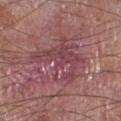This lesion was catalogued during total-body skin photography and was not selected for biopsy.
Automated tile analysis of the lesion measured an area of roughly 19 mm², a shape eccentricity near 0.65, and a symmetry-axis asymmetry near 0.45. The analysis additionally found a within-lesion color-variation index near 5/10. The software also gave an automated nevus-likeness rating near 0 out of 100 and a detector confidence of about 55 out of 100 that the crop contains a lesion.
A male subject aged around 60.
This is a white-light tile.
The lesion is located on the left lower leg.
Longest diameter approximately 6.5 mm.
This image is a 15 mm lesion crop taken from a total-body photograph.